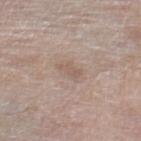follow-up — total-body-photography surveillance lesion; no biopsy | site — the right thigh | acquisition — 15 mm crop, total-body photography | automated lesion analysis — an eccentricity of roughly 0.85 and a shape-asymmetry score of about 0.35 (0 = symmetric); a mean CIELAB color near L≈57 a*≈15 b*≈24, a lesion–skin lightness drop of about 6, and a normalized border contrast of about 4.5; internal color variation of about 1 on a 0–10 scale and a peripheral color-asymmetry measure near 0.5; an automated nevus-likeness rating near 0 out of 100 and a lesion-detection confidence of about 100/100 | patient — male, aged 78 to 82 | lesion diameter — ~3 mm (longest diameter) | illumination — white-light.